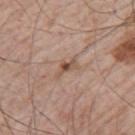Assessment: Imaged during a routine full-body skin examination; the lesion was not biopsied and no histopathology is available. Image and clinical context: A male patient, roughly 65 years of age. From the left upper arm. A region of skin cropped from a whole-body photographic capture, roughly 15 mm wide.